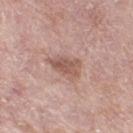<lesion>
  <biopsy_status>not biopsied; imaged during a skin examination</biopsy_status>
  <automated_metrics>
    <area_mm2_approx>7.5</area_mm2_approx>
    <eccentricity>0.8</eccentricity>
    <shape_asymmetry>0.3</shape_asymmetry>
  </automated_metrics>
  <patient>
    <sex>female</sex>
    <age_approx>70</age_approx>
  </patient>
  <image>
    <source>total-body photography crop</source>
    <field_of_view_mm>15</field_of_view_mm>
  </image>
  <lesion_size>
    <long_diameter_mm_approx>4.0</long_diameter_mm_approx>
  </lesion_size>
  <site>right thigh</site>
</lesion>